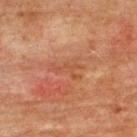{
  "biopsy_status": "not biopsied; imaged during a skin examination",
  "automated_metrics": {
    "color_variation_0_10": 2.0,
    "peripheral_color_asymmetry": 0.5
  },
  "patient": {
    "sex": "male",
    "age_approx": 75
  },
  "lighting": "cross-polarized",
  "site": "upper back",
  "lesion_size": {
    "long_diameter_mm_approx": 4.0
  },
  "image": {
    "source": "total-body photography crop",
    "field_of_view_mm": 15
  }
}